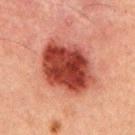| key | value |
|---|---|
| biopsy status | total-body-photography surveillance lesion; no biopsy |
| image | total-body-photography crop, ~15 mm field of view |
| patient | male, approximately 50 years of age |
| lighting | cross-polarized |
| body site | the upper back |